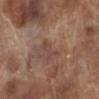Captured during whole-body skin photography for melanoma surveillance; the lesion was not biopsied. The subject is a male approximately 70 years of age. Automated tile analysis of the lesion measured a lesion color around L≈45 a*≈20 b*≈25 in CIELAB, about 6 CIELAB-L* units darker than the surrounding skin, and a normalized lesion–skin contrast near 5. The analysis additionally found border irregularity of about 5.5 on a 0–10 scale, internal color variation of about 0 on a 0–10 scale, and radial color variation of about 0. The analysis additionally found an automated nevus-likeness rating near 0 out of 100 and a lesion-detection confidence of about 100/100. The recorded lesion diameter is about 3.5 mm. Imaged with white-light lighting. A roughly 15 mm field-of-view crop from a total-body skin photograph. The lesion is located on the left lower leg.